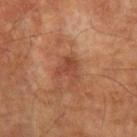Assessment: The lesion was photographed on a routine skin check and not biopsied; there is no pathology result. Background: A lesion tile, about 15 mm wide, cut from a 3D total-body photograph. The lesion-visualizer software estimated an automated nevus-likeness rating near 0 out of 100. Longest diameter approximately 2.5 mm. The lesion is located on the left upper arm. A male patient aged 63–67. This is a cross-polarized tile.